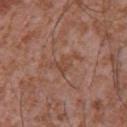Notes:
* acquisition: ~15 mm crop, total-body skin-cancer survey
* anatomic site: the chest
* subject: male, about 45 years old